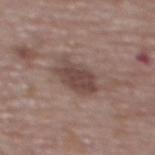Q: Is there a histopathology result?
A: catalogued during a skin exam; not biopsied
Q: What kind of image is this?
A: total-body-photography crop, ~15 mm field of view
Q: Who is the patient?
A: female, roughly 65 years of age
Q: Lesion location?
A: the back